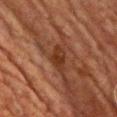follow-up — no biopsy performed (imaged during a skin exam) | subject — male, aged around 60 | image-analysis metrics — a lesion area of about 5 mm², an eccentricity of roughly 0.85, and a shape-asymmetry score of about 0.3 (0 = symmetric); a lesion color around L≈26 a*≈19 b*≈26 in CIELAB, roughly 7 lightness units darker than nearby skin, and a normalized lesion–skin contrast near 8; a border-irregularity index near 3.5/10, a within-lesion color-variation index near 2/10, and radial color variation of about 0.5 | lesion diameter — about 3.5 mm | site — the chest | imaging modality — ~15 mm tile from a whole-body skin photo.